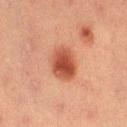Recorded during total-body skin imaging; not selected for excision or biopsy.
On the right thigh.
The recorded lesion diameter is about 4 mm.
Imaged with cross-polarized lighting.
A female patient in their mid-50s.
Cropped from a whole-body photographic skin survey; the tile spans about 15 mm.